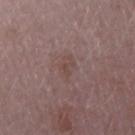Clinical impression:
Captured during whole-body skin photography for melanoma surveillance; the lesion was not biopsied.
Background:
Located on the right upper arm. Automated image analysis of the tile measured a border-irregularity index near 4/10 and a within-lesion color-variation index near 2/10. The software also gave an automated nevus-likeness rating near 0 out of 100. A 15 mm crop from a total-body photograph taken for skin-cancer surveillance. Imaged with white-light lighting. The subject is a female aged approximately 45. Longest diameter approximately 3.5 mm.